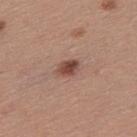image = total-body-photography crop, ~15 mm field of view | anatomic site = the upper back | image-analysis metrics = an area of roughly 4.5 mm² and a symmetry-axis asymmetry near 0.2; a border-irregularity rating of about 1.5/10, internal color variation of about 3.5 on a 0–10 scale, and radial color variation of about 1.5; a nevus-likeness score of about 95/100 and lesion-presence confidence of about 100/100 | tile lighting = white-light | patient = male, aged 53–57 | diameter = ≈2.5 mm.